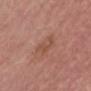| key | value |
|---|---|
| follow-up | total-body-photography surveillance lesion; no biopsy |
| patient | male, aged approximately 70 |
| image | total-body-photography crop, ~15 mm field of view |
| anatomic site | the front of the torso |